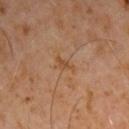follow-up: imaged on a skin check; not biopsied
body site: the arm
diameter: about 2.5 mm
imaging modality: total-body-photography crop, ~15 mm field of view
subject: male, roughly 60 years of age
automated metrics: an average lesion color of about L≈45 a*≈19 b*≈33 (CIELAB), about 6 CIELAB-L* units darker than the surrounding skin, and a lesion-to-skin contrast of about 6 (normalized; higher = more distinct); an automated nevus-likeness rating near 0 out of 100 and a detector confidence of about 100 out of 100 that the crop contains a lesion
illumination: cross-polarized illumination